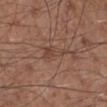This lesion was catalogued during total-body skin photography and was not selected for biopsy. A lesion tile, about 15 mm wide, cut from a 3D total-body photograph. The lesion is located on the right lower leg. A male patient aged 58 to 62.